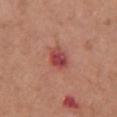Q: Was a biopsy performed?
A: catalogued during a skin exam; not biopsied